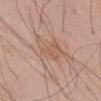workup — total-body-photography surveillance lesion; no biopsy
patient — male, aged 58 to 62
anatomic site — the chest
image source — total-body-photography crop, ~15 mm field of view
illumination — white-light